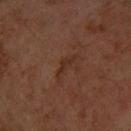Findings:
* patient: female, roughly 60 years of age
* tile lighting: cross-polarized illumination
* acquisition: ~15 mm crop, total-body skin-cancer survey
* anatomic site: the arm
* lesion size: ≈3.5 mm